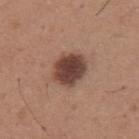Q: Was this lesion biopsied?
A: total-body-photography surveillance lesion; no biopsy
Q: What is the anatomic site?
A: the upper back
Q: Patient demographics?
A: male, aged approximately 65
Q: How was this image acquired?
A: total-body-photography crop, ~15 mm field of view
Q: How was the tile lit?
A: white-light illumination
Q: How large is the lesion?
A: ~4 mm (longest diameter)
Q: Automated lesion metrics?
A: radial color variation of about 1